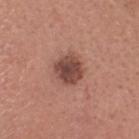Imaged during a routine full-body skin examination; the lesion was not biopsied and no histopathology is available.
On the head or neck.
An algorithmic analysis of the crop reported an average lesion color of about L≈45 a*≈22 b*≈24 (CIELAB), a lesion–skin lightness drop of about 14, and a lesion-to-skin contrast of about 10 (normalized; higher = more distinct). The software also gave a classifier nevus-likeness of about 45/100 and a lesion-detection confidence of about 100/100.
A male patient, aged 38 to 42.
Captured under white-light illumination.
A 15 mm crop from a total-body photograph taken for skin-cancer surveillance.
Measured at roughly 3 mm in maximum diameter.